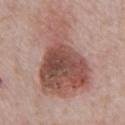Impression:
Part of a total-body skin-imaging series; this lesion was reviewed on a skin check and was not flagged for biopsy.
Context:
This is a white-light tile. Located on the abdomen. A male subject, about 70 years old. A close-up tile cropped from a whole-body skin photograph, about 15 mm across.